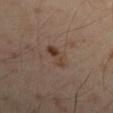<lesion>
<biopsy_status>not biopsied; imaged during a skin examination</biopsy_status>
<lighting>cross-polarized</lighting>
<patient>
  <sex>male</sex>
  <age_approx>50</age_approx>
</patient>
<image>
  <source>total-body photography crop</source>
  <field_of_view_mm>15</field_of_view_mm>
</image>
<site>left upper arm</site>
<lesion_size>
  <long_diameter_mm_approx>3.5</long_diameter_mm_approx>
</lesion_size>
</lesion>